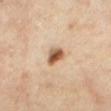No biopsy was performed on this lesion — it was imaged during a full skin examination and was not determined to be concerning. Located on the mid back. A female patient aged around 60. The total-body-photography lesion software estimated a classifier nevus-likeness of about 100/100 and lesion-presence confidence of about 100/100. Captured under cross-polarized illumination. Cropped from a total-body skin-imaging series; the visible field is about 15 mm. The lesion's longest dimension is about 3 mm.